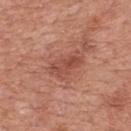Acquisition and patient details:
A 15 mm close-up tile from a total-body photography series done for melanoma screening. Measured at roughly 4 mm in maximum diameter. A male subject, approximately 70 years of age. The lesion is on the mid back.